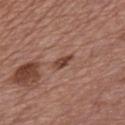The lesion was tiled from a total-body skin photograph and was not biopsied.
Measured at roughly 2.5 mm in maximum diameter.
A close-up tile cropped from a whole-body skin photograph, about 15 mm across.
The lesion is on the chest.
A male patient, in their 70s.